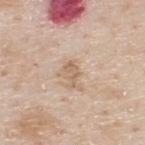Findings:
- biopsy status: no biopsy performed (imaged during a skin exam)
- diameter: ≈3 mm
- body site: the upper back
- patient: male, roughly 80 years of age
- acquisition: 15 mm crop, total-body photography
- lighting: white-light
- automated lesion analysis: an eccentricity of roughly 0.85 and two-axis asymmetry of about 0.45; an average lesion color of about L≈62 a*≈16 b*≈31 (CIELAB) and a normalized border contrast of about 6.5; border irregularity of about 5 on a 0–10 scale; a nevus-likeness score of about 0/100